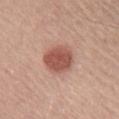* biopsy status — catalogued during a skin exam; not biopsied
* subject — male, aged 63 to 67
* image source — total-body-photography crop, ~15 mm field of view
* location — the upper back